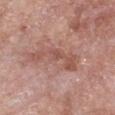| feature | finding |
|---|---|
| biopsy status | no biopsy performed (imaged during a skin exam) |
| TBP lesion metrics | an average lesion color of about L≈53 a*≈23 b*≈26 (CIELAB), a lesion–skin lightness drop of about 9, and a normalized lesion–skin contrast near 6; an automated nevus-likeness rating near 0 out of 100 |
| image source | 15 mm crop, total-body photography |
| patient | female, aged approximately 75 |
| illumination | white-light |
| body site | the chest |
| size | ~6.5 mm (longest diameter) |